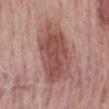Notes:
– follow-up — catalogued during a skin exam; not biopsied
– tile lighting — white-light
– patient — male, aged approximately 50
– site — the mid back
– image-analysis metrics — an area of roughly 29 mm², an outline eccentricity of about 0.8 (0 = round, 1 = elongated), and a symmetry-axis asymmetry near 0.15; a lesion color around L≈50 a*≈23 b*≈23 in CIELAB and roughly 11 lightness units darker than nearby skin; border irregularity of about 2.5 on a 0–10 scale, internal color variation of about 4.5 on a 0–10 scale, and radial color variation of about 1.5
– image — ~15 mm tile from a whole-body skin photo
– lesion size — ≈8 mm